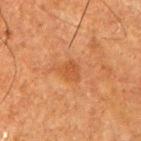| key | value |
|---|---|
| biopsy status | total-body-photography surveillance lesion; no biopsy |
| subject | male, aged 73 to 77 |
| automated metrics | an area of roughly 4 mm², an outline eccentricity of about 0.55 (0 = round, 1 = elongated), and a shape-asymmetry score of about 0.3 (0 = symmetric); internal color variation of about 1.5 on a 0–10 scale and peripheral color asymmetry of about 0.5 |
| lesion size | about 2.5 mm |
| site | the right upper arm |
| illumination | cross-polarized |
| acquisition | 15 mm crop, total-body photography |